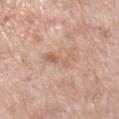- notes — imaged on a skin check; not biopsied
- imaging modality — total-body-photography crop, ~15 mm field of view
- subject — male, aged 68–72
- anatomic site — the left upper arm